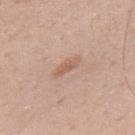{"biopsy_status": "not biopsied; imaged during a skin examination", "image": {"source": "total-body photography crop", "field_of_view_mm": 15}, "patient": {"sex": "male", "age_approx": 70}, "lighting": "white-light", "site": "upper back", "automated_metrics": {"border_irregularity_0_10": 3.5, "color_variation_0_10": 0.5, "peripheral_color_asymmetry": 0.0, "nevus_likeness_0_100": 0, "lesion_detection_confidence_0_100": 100}}